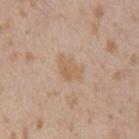Notes:
– follow-up · total-body-photography surveillance lesion; no biopsy
– image · total-body-photography crop, ~15 mm field of view
– site · the left upper arm
– subject · male, aged approximately 25